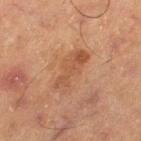Case summary:
* notes — imaged on a skin check; not biopsied
* patient — male, about 65 years old
* TBP lesion metrics — a footprint of about 12 mm², a shape eccentricity near 0.9, and a shape-asymmetry score of about 0.2 (0 = symmetric); a border-irregularity rating of about 3/10, a color-variation rating of about 3.5/10, and a peripheral color-asymmetry measure near 1; a nevus-likeness score of about 0/100 and a lesion-detection confidence of about 100/100
* tile lighting — cross-polarized
* diameter — ≈5.5 mm
* site — the right thigh
* acquisition — total-body-photography crop, ~15 mm field of view